A female patient in their 50s; located on the left thigh; the lesion's longest dimension is about 2.5 mm; cropped from a total-body skin-imaging series; the visible field is about 15 mm; captured under cross-polarized illumination: 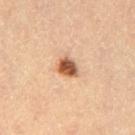Pathology: The biopsy diagnosis was an atypical melanocytic neoplasm — a lesion of indeterminate malignant potential.